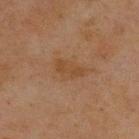The lesion was photographed on a routine skin check and not biopsied; there is no pathology result. Longest diameter approximately 3.5 mm. This is a cross-polarized tile. A 15 mm crop from a total-body photograph taken for skin-cancer surveillance. On the upper back. The subject is a male aged 43–47.